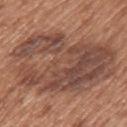biopsy status = imaged on a skin check; not biopsied
acquisition = 15 mm crop, total-body photography
site = the back
patient = male, roughly 65 years of age
diameter = ≈12 mm